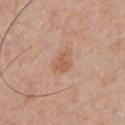Case summary:
– notes · catalogued during a skin exam; not biopsied
– subject · male, aged around 60
– diameter · ~3 mm (longest diameter)
– acquisition · ~15 mm tile from a whole-body skin photo
– body site · the chest